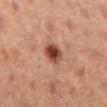Clinical impression:
Part of a total-body skin-imaging series; this lesion was reviewed on a skin check and was not flagged for biopsy.
Clinical summary:
A 15 mm close-up tile from a total-body photography series done for melanoma screening. The lesion is on the right lower leg. This is a cross-polarized tile. Approximately 3 mm at its widest. A female subject, about 40 years old.